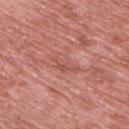Located on the upper back. A male subject, roughly 70 years of age. Cropped from a total-body skin-imaging series; the visible field is about 15 mm. Measured at roughly 3.5 mm in maximum diameter. Imaged with white-light lighting.